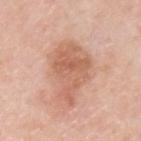Captured during whole-body skin photography for melanoma surveillance; the lesion was not biopsied. Located on the upper back. The tile uses white-light illumination. Measured at roughly 6.5 mm in maximum diameter. A roughly 15 mm field-of-view crop from a total-body skin photograph. A male subject, about 60 years old.